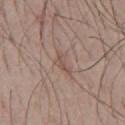Captured during whole-body skin photography for melanoma surveillance; the lesion was not biopsied.
The subject is a male in their mid-60s.
The lesion is located on the chest.
This image is a 15 mm lesion crop taken from a total-body photograph.